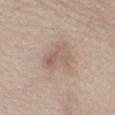Recorded during total-body skin imaging; not selected for excision or biopsy.
On the leg.
Imaged with white-light lighting.
A region of skin cropped from a whole-body photographic capture, roughly 15 mm wide.
The subject is a female aged 58–62.
The lesion-visualizer software estimated border irregularity of about 4.5 on a 0–10 scale, a color-variation rating of about 4.5/10, and radial color variation of about 1.5. It also reported a nevus-likeness score of about 60/100 and lesion-presence confidence of about 100/100.